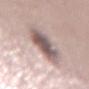{
  "biopsy_status": "not biopsied; imaged during a skin examination"
}